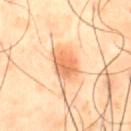Findings:
• biopsy status — no biopsy performed (imaged during a skin exam)
• lesion size — ~3.5 mm (longest diameter)
• TBP lesion metrics — radial color variation of about 1
• subject — male, in their 50s
• image — ~15 mm crop, total-body skin-cancer survey
• site — the front of the torso
• tile lighting — cross-polarized